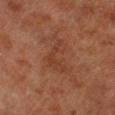Captured during whole-body skin photography for melanoma surveillance; the lesion was not biopsied. Captured under cross-polarized illumination. A male subject approximately 80 years of age. About 5.5 mm across. The lesion is located on the right lower leg. An algorithmic analysis of the crop reported an eccentricity of roughly 0.8 and a symmetry-axis asymmetry near 0.6. It also reported a lesion–skin lightness drop of about 4 and a normalized border contrast of about 4.5. And it measured border irregularity of about 8 on a 0–10 scale. A 15 mm crop from a total-body photograph taken for skin-cancer surveillance.